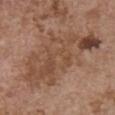Impression:
The lesion was photographed on a routine skin check and not biopsied; there is no pathology result.
Acquisition and patient details:
About 11 mm across. Imaged with white-light lighting. A region of skin cropped from a whole-body photographic capture, roughly 15 mm wide. On the chest. The patient is a female aged 63–67.